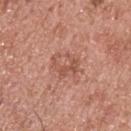Captured during whole-body skin photography for melanoma surveillance; the lesion was not biopsied.
A lesion tile, about 15 mm wide, cut from a 3D total-body photograph.
The tile uses white-light illumination.
A male patient, aged around 55.
From the upper back.